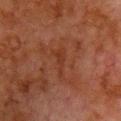Imaged during a routine full-body skin examination; the lesion was not biopsied and no histopathology is available.
The lesion is on the chest.
Automated tile analysis of the lesion measured an area of roughly 3.5 mm², an outline eccentricity of about 0.95 (0 = round, 1 = elongated), and a symmetry-axis asymmetry near 0.5. And it measured a mean CIELAB color near L≈27 a*≈21 b*≈26, roughly 4 lightness units darker than nearby skin, and a normalized lesion–skin contrast near 5.5. It also reported a color-variation rating of about 0.5/10. The analysis additionally found a nevus-likeness score of about 0/100 and a detector confidence of about 85 out of 100 that the crop contains a lesion.
Imaged with cross-polarized lighting.
A roughly 15 mm field-of-view crop from a total-body skin photograph.
The subject is a male aged around 80.
Longest diameter approximately 3.5 mm.